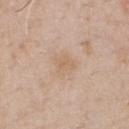Q: How large is the lesion?
A: ≈3 mm
Q: Lesion location?
A: the front of the torso
Q: What is the imaging modality?
A: ~15 mm crop, total-body skin-cancer survey
Q: What are the patient's age and sex?
A: male, aged 48–52
Q: What did automated image analysis measure?
A: a lesion color around L≈64 a*≈16 b*≈31 in CIELAB, roughly 5 lightness units darker than nearby skin, and a normalized lesion–skin contrast near 4.5; border irregularity of about 3 on a 0–10 scale
Q: Illumination type?
A: white-light illumination